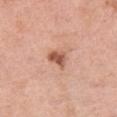Case summary:
* workup · total-body-photography surveillance lesion; no biopsy
* lesion diameter · ≈3 mm
* automated lesion analysis · roughly 14 lightness units darker than nearby skin and a normalized lesion–skin contrast near 8.5; internal color variation of about 3.5 on a 0–10 scale and radial color variation of about 1
* location · the left thigh
* illumination · white-light
* image source · ~15 mm tile from a whole-body skin photo
* patient · female, aged 53 to 57